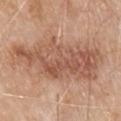workup: no biopsy performed (imaged during a skin exam)
acquisition: ~15 mm crop, total-body skin-cancer survey
image-analysis metrics: a nevus-likeness score of about 0/100
size: ≈12.5 mm
location: the chest
lighting: white-light illumination
patient: male, aged 78 to 82